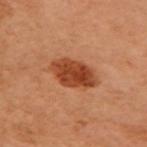Notes:
• image source · ~15 mm tile from a whole-body skin photo
• lighting · cross-polarized illumination
• size · ~5 mm (longest diameter)
• automated lesion analysis · a shape eccentricity near 0.75 and a symmetry-axis asymmetry near 0.15; a lesion–skin lightness drop of about 12 and a normalized lesion–skin contrast near 10; a border-irregularity rating of about 2/10, internal color variation of about 4 on a 0–10 scale, and peripheral color asymmetry of about 1.5; lesion-presence confidence of about 100/100
• anatomic site · the arm
• subject · female, aged 58–62